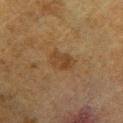The lesion was tiled from a total-body skin photograph and was not biopsied.
The total-body-photography lesion software estimated a footprint of about 6.5 mm² and a shape-asymmetry score of about 0.2 (0 = symmetric). The software also gave an average lesion color of about L≈32 a*≈14 b*≈27 (CIELAB), about 6 CIELAB-L* units darker than the surrounding skin, and a lesion-to-skin contrast of about 6.5 (normalized; higher = more distinct). The analysis additionally found peripheral color asymmetry of about 1. The software also gave an automated nevus-likeness rating near 30 out of 100 and a lesion-detection confidence of about 100/100.
Approximately 3.5 mm at its widest.
The tile uses cross-polarized illumination.
The lesion is located on the leg.
A close-up tile cropped from a whole-body skin photograph, about 15 mm across.
The patient is a male approximately 60 years of age.